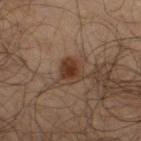| field | value |
|---|---|
| site | the right thigh |
| lighting | cross-polarized illumination |
| TBP lesion metrics | two-axis asymmetry of about 0.35; an average lesion color of about L≈33 a*≈18 b*≈26 (CIELAB) and about 10 CIELAB-L* units darker than the surrounding skin; a border-irregularity index near 3.5/10, a within-lesion color-variation index near 2.5/10, and peripheral color asymmetry of about 0.5; a nevus-likeness score of about 90/100 and a lesion-detection confidence of about 100/100 |
| subject | male, aged approximately 65 |
| acquisition | ~15 mm tile from a whole-body skin photo |
| diameter | about 3 mm |